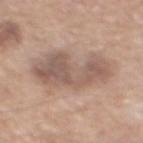Assessment:
This lesion was catalogued during total-body skin photography and was not selected for biopsy.
Background:
This is a white-light tile. An algorithmic analysis of the crop reported an area of roughly 24 mm², an outline eccentricity of about 0.8 (0 = round, 1 = elongated), and a symmetry-axis asymmetry near 0.35. The analysis additionally found border irregularity of about 4.5 on a 0–10 scale and peripheral color asymmetry of about 1.5. The software also gave a nevus-likeness score of about 0/100 and a lesion-detection confidence of about 100/100. From the chest. A male patient, aged 68 to 72. Cropped from a total-body skin-imaging series; the visible field is about 15 mm.